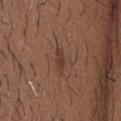notes: no biopsy performed (imaged during a skin exam) | anatomic site: the head or neck | acquisition: ~15 mm tile from a whole-body skin photo | subject: male, aged 23–27.